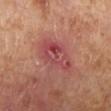follow-up: total-body-photography surveillance lesion; no biopsy | lesion size: ≈4.5 mm | image: ~15 mm crop, total-body skin-cancer survey | anatomic site: the right lower leg | automated lesion analysis: border irregularity of about 2.5 on a 0–10 scale and a color-variation rating of about 8.5/10 | lighting: cross-polarized | subject: female, approximately 55 years of age.